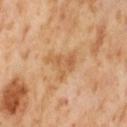subject=female, aged around 55 | automated lesion analysis=an average lesion color of about L≈60 a*≈22 b*≈40 (CIELAB), a lesion–skin lightness drop of about 8, and a normalized border contrast of about 6 | lesion size=~4 mm (longest diameter) | anatomic site=the right thigh | imaging modality=total-body-photography crop, ~15 mm field of view.